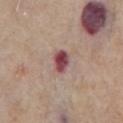workup = no biopsy performed (imaged during a skin exam); patient = male, approximately 65 years of age; body site = the chest; size = ≈3 mm; illumination = white-light; acquisition = 15 mm crop, total-body photography.